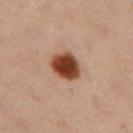The lesion was tiled from a total-body skin photograph and was not biopsied. A lesion tile, about 15 mm wide, cut from a 3D total-body photograph. A female subject, aged approximately 40. The recorded lesion diameter is about 4 mm. This is a cross-polarized tile. On the left thigh.